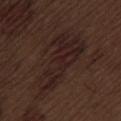Clinical impression: The lesion was photographed on a routine skin check and not biopsied; there is no pathology result. Clinical summary: A male patient aged 68–72. This is a white-light tile. From the lower back. A close-up tile cropped from a whole-body skin photograph, about 15 mm across.